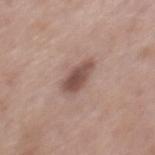Cropped from a total-body skin-imaging series; the visible field is about 15 mm. A male subject, in their mid-50s. The recorded lesion diameter is about 4 mm. On the mid back. Automated image analysis of the tile measured an area of roughly 7 mm², an eccentricity of roughly 0.8, and two-axis asymmetry of about 0.2. And it measured a lesion color around L≈50 a*≈18 b*≈23 in CIELAB and a normalized lesion–skin contrast near 9. The analysis additionally found border irregularity of about 2 on a 0–10 scale, a within-lesion color-variation index near 3.5/10, and radial color variation of about 1. It also reported an automated nevus-likeness rating near 70 out of 100.